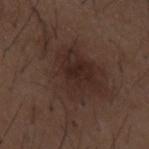Q: Was this lesion biopsied?
A: total-body-photography surveillance lesion; no biopsy
Q: Lesion size?
A: ≈12 mm
Q: Lesion location?
A: the mid back
Q: Automated lesion metrics?
A: border irregularity of about 8.5 on a 0–10 scale, a color-variation rating of about 4/10, and radial color variation of about 1.5; an automated nevus-likeness rating near 75 out of 100 and a detector confidence of about 100 out of 100 that the crop contains a lesion
Q: Who is the patient?
A: male, roughly 50 years of age
Q: What is the imaging modality?
A: ~15 mm crop, total-body skin-cancer survey
Q: What lighting was used for the tile?
A: white-light illumination